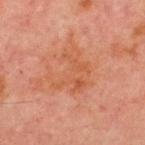– workup · total-body-photography surveillance lesion; no biopsy
– site · the chest
– tile lighting · cross-polarized
– lesion diameter · about 5.5 mm
– subject · male, about 70 years old
– image · ~15 mm crop, total-body skin-cancer survey